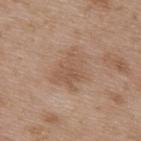Part of a total-body skin-imaging series; this lesion was reviewed on a skin check and was not flagged for biopsy. The lesion is on the back. The total-body-photography lesion software estimated an eccentricity of roughly 0.65 and two-axis asymmetry of about 0.45. And it measured a classifier nevus-likeness of about 0/100 and a lesion-detection confidence of about 100/100. The patient is a male aged approximately 50. A lesion tile, about 15 mm wide, cut from a 3D total-body photograph.